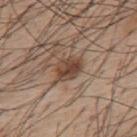About 3.5 mm across.
On the upper back.
This is a white-light tile.
A male patient, aged 53–57.
A 15 mm close-up extracted from a 3D total-body photography capture.